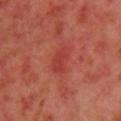Imaged during a routine full-body skin examination; the lesion was not biopsied and no histopathology is available.
Cropped from a whole-body photographic skin survey; the tile spans about 15 mm.
A female patient, about 40 years old.
Automated image analysis of the tile measured roughly 6 lightness units darker than nearby skin. And it measured border irregularity of about 3 on a 0–10 scale, a within-lesion color-variation index near 1.5/10, and radial color variation of about 0.5.
The lesion is on the left upper arm.
The lesion's longest dimension is about 3 mm.
Imaged with cross-polarized lighting.